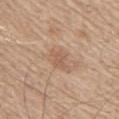biopsy status: imaged on a skin check; not biopsied
patient: male, about 65 years old
anatomic site: the right upper arm
diameter: ≈2.5 mm
acquisition: 15 mm crop, total-body photography
lighting: white-light illumination